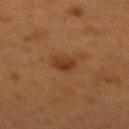illumination=cross-polarized
TBP lesion metrics=a lesion area of about 4.5 mm²; an average lesion color of about L≈29 a*≈20 b*≈27 (CIELAB), about 7 CIELAB-L* units darker than the surrounding skin, and a normalized border contrast of about 7.5
size=~2.5 mm (longest diameter)
image=15 mm crop, total-body photography
site=the mid back
subject=female, roughly 50 years of age